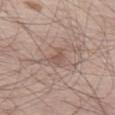- follow-up · no biopsy performed (imaged during a skin exam)
- acquisition · total-body-photography crop, ~15 mm field of view
- subject · male, in their mid- to late 60s
- tile lighting · white-light illumination
- site · the left thigh
- diameter · about 2.5 mm